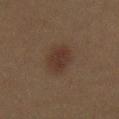Q: Is there a histopathology result?
A: imaged on a skin check; not biopsied
Q: What lighting was used for the tile?
A: cross-polarized illumination
Q: What is the imaging modality?
A: 15 mm crop, total-body photography
Q: What is the lesion's diameter?
A: ~4 mm (longest diameter)
Q: Who is the patient?
A: male, aged around 50
Q: Lesion location?
A: the front of the torso
Q: What did automated image analysis measure?
A: a footprint of about 6.5 mm², a shape eccentricity near 0.85, and a symmetry-axis asymmetry near 0.2; a border-irregularity index near 2/10, internal color variation of about 2 on a 0–10 scale, and peripheral color asymmetry of about 1